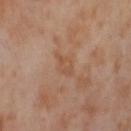| field | value |
|---|---|
| follow-up | total-body-photography surveillance lesion; no biopsy |
| subject | female, roughly 55 years of age |
| anatomic site | the right thigh |
| illumination | cross-polarized |
| image source | ~15 mm tile from a whole-body skin photo |